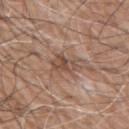<tbp_lesion>
  <biopsy_status>not biopsied; imaged during a skin examination</biopsy_status>
  <site>arm</site>
  <lesion_size>
    <long_diameter_mm_approx>4.0</long_diameter_mm_approx>
  </lesion_size>
  <lighting>white-light</lighting>
  <patient>
    <sex>male</sex>
    <age_approx>60</age_approx>
  </patient>
  <image>
    <source>total-body photography crop</source>
    <field_of_view_mm>15</field_of_view_mm>
  </image>
</tbp_lesion>